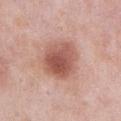Notes:
• notes · catalogued during a skin exam; not biopsied
• subject · male, aged around 55
• image source · 15 mm crop, total-body photography
• location · the abdomen